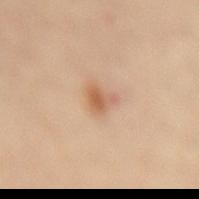{"biopsy_status": "not biopsied; imaged during a skin examination", "site": "lower back", "image": {"source": "total-body photography crop", "field_of_view_mm": 15}, "lighting": "cross-polarized", "patient": {"sex": "female", "age_approx": 60}, "lesion_size": {"long_diameter_mm_approx": 2.5}}